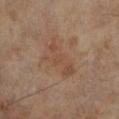Recorded during total-body skin imaging; not selected for excision or biopsy. Located on the right lower leg. The patient is a female in their mid-70s. A 15 mm close-up tile from a total-body photography series done for melanoma screening.